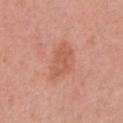Q: Illumination type?
A: white-light
Q: Where on the body is the lesion?
A: the right upper arm
Q: Automated lesion metrics?
A: a lesion area of about 11 mm² and two-axis asymmetry of about 0.3; a nevus-likeness score of about 5/100 and a lesion-detection confidence of about 100/100
Q: Lesion size?
A: about 4.5 mm
Q: Patient demographics?
A: female, aged 53–57
Q: What is the imaging modality?
A: total-body-photography crop, ~15 mm field of view